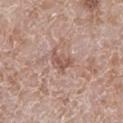Recorded during total-body skin imaging; not selected for excision or biopsy.